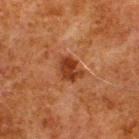illumination — cross-polarized illumination | site — the back | diameter — about 3 mm | automated metrics — roughly 9 lightness units darker than nearby skin and a normalized border contrast of about 9; a border-irregularity rating of about 3/10 and a color-variation rating of about 3/10; an automated nevus-likeness rating near 90 out of 100 and a lesion-detection confidence of about 100/100 | patient — male, in their 80s | imaging modality — ~15 mm crop, total-body skin-cancer survey.